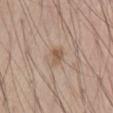  biopsy_status: not biopsied; imaged during a skin examination
  automated_metrics:
    area_mm2_approx: 4.0
    eccentricity: 0.85
    shape_asymmetry: 0.3
    cielab_L: 56
    cielab_a: 16
    cielab_b: 29
    vs_skin_contrast_norm: 6.5
    border_irregularity_0_10: 3.0
    peripheral_color_asymmetry: 1.0
    nevus_likeness_0_100: 5
    lesion_detection_confidence_0_100: 100
  patient:
    sex: male
    age_approx: 50
  image:
    source: total-body photography crop
    field_of_view_mm: 15
  site: left upper arm
  lesion_size:
    long_diameter_mm_approx: 3.0
  lighting: white-light